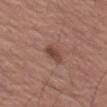{
  "biopsy_status": "not biopsied; imaged during a skin examination",
  "lesion_size": {
    "long_diameter_mm_approx": 3.0
  },
  "patient": {
    "sex": "male",
    "age_approx": 65
  },
  "lighting": "white-light",
  "site": "left thigh",
  "image": {
    "source": "total-body photography crop",
    "field_of_view_mm": 15
  }
}